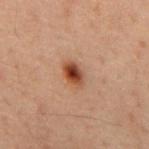lesion diameter — ≈3 mm
subject — male, roughly 60 years of age
body site — the mid back
TBP lesion metrics — border irregularity of about 1.5 on a 0–10 scale and a peripheral color-asymmetry measure near 1.5
tile lighting — cross-polarized illumination
image — ~15 mm tile from a whole-body skin photo